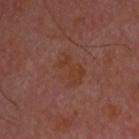Clinical impression:
Imaged during a routine full-body skin examination; the lesion was not biopsied and no histopathology is available.
Context:
The lesion's longest dimension is about 4.5 mm. Located on the head or neck. A male subject, in their mid-60s. Cropped from a total-body skin-imaging series; the visible field is about 15 mm. Imaged with cross-polarized lighting.